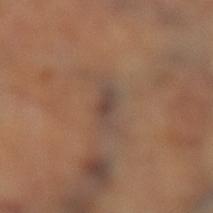<case>
  <biopsy_status>not biopsied; imaged during a skin examination</biopsy_status>
  <image>
    <source>total-body photography crop</source>
    <field_of_view_mm>15</field_of_view_mm>
  </image>
  <lesion_size>
    <long_diameter_mm_approx>3.5</long_diameter_mm_approx>
  </lesion_size>
  <site>left lower leg</site>
</case>